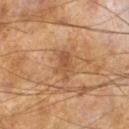Image and clinical context:
A close-up tile cropped from a whole-body skin photograph, about 15 mm across. A male patient approximately 65 years of age. The recorded lesion diameter is about 3.5 mm. The tile uses cross-polarized illumination.